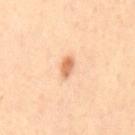Part of a total-body skin-imaging series; this lesion was reviewed on a skin check and was not flagged for biopsy. The lesion's longest dimension is about 2.5 mm. A region of skin cropped from a whole-body photographic capture, roughly 15 mm wide. From the back. This is a cross-polarized tile. A male subject, approximately 45 years of age.